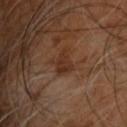This lesion was catalogued during total-body skin photography and was not selected for biopsy. From the upper back. The lesion's longest dimension is about 2.5 mm. This is a cross-polarized tile. A 15 mm close-up extracted from a 3D total-body photography capture. A male subject, in their 60s.